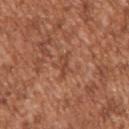| feature | finding |
|---|---|
| image source | 15 mm crop, total-body photography |
| anatomic site | the right upper arm |
| lesion size | ~3 mm (longest diameter) |
| subject | male, aged around 45 |
| automated metrics | an area of roughly 3 mm², a shape eccentricity near 0.9, and a symmetry-axis asymmetry near 0.35; a mean CIELAB color near L≈46 a*≈24 b*≈32, roughly 7 lightness units darker than nearby skin, and a normalized lesion–skin contrast near 5.5; a lesion-detection confidence of about 85/100 |